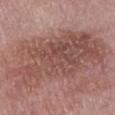No biopsy was performed on this lesion — it was imaged during a full skin examination and was not determined to be concerning. A region of skin cropped from a whole-body photographic capture, roughly 15 mm wide. The subject is a male in their mid-70s. The recorded lesion diameter is about 14 mm. Imaged with white-light lighting. The lesion is on the front of the torso.